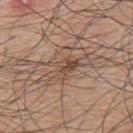The lesion was tiled from a total-body skin photograph and was not biopsied.
A 15 mm close-up tile from a total-body photography series done for melanoma screening.
This is a white-light tile.
The lesion is on the mid back.
A male patient, about 75 years old.
The lesion-visualizer software estimated a lesion area of about 5.5 mm². The software also gave border irregularity of about 6 on a 0–10 scale, a color-variation rating of about 4.5/10, and radial color variation of about 2. The analysis additionally found a classifier nevus-likeness of about 0/100 and lesion-presence confidence of about 95/100.
Approximately 3.5 mm at its widest.